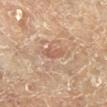Case summary:
* notes · catalogued during a skin exam; not biopsied
* imaging modality · ~15 mm tile from a whole-body skin photo
* automated lesion analysis · a mean CIELAB color near L≈56 a*≈20 b*≈30 and a normalized border contrast of about 5.5
* lighting · cross-polarized
* location · the leg
* patient · male, in their mid- to late 80s or older
* lesion size · about 3 mm